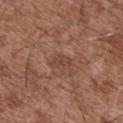A male subject in their mid-70s. A lesion tile, about 15 mm wide, cut from a 3D total-body photograph. On the chest.